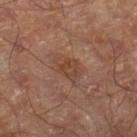Findings:
– workup — no biopsy performed (imaged during a skin exam)
– illumination — cross-polarized
– location — the right thigh
– image — 15 mm crop, total-body photography
– diameter — about 4 mm